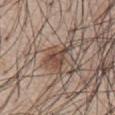- follow-up · no biopsy performed (imaged during a skin exam)
- anatomic site · the chest
- lighting · white-light illumination
- subject · male, aged around 55
- acquisition · 15 mm crop, total-body photography
- automated lesion analysis · border irregularity of about 6 on a 0–10 scale, a color-variation rating of about 3.5/10, and radial color variation of about 1; a nevus-likeness score of about 90/100